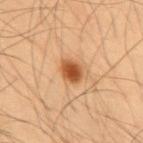Notes:
- biopsy status — no biopsy performed (imaged during a skin exam)
- patient — male, aged around 55
- size — about 2.5 mm
- illumination — cross-polarized illumination
- automated metrics — an outline eccentricity of about 0.6 (0 = round, 1 = elongated) and a shape-asymmetry score of about 0.15 (0 = symmetric); a classifier nevus-likeness of about 100/100 and a lesion-detection confidence of about 100/100
- site — the mid back
- imaging modality — total-body-photography crop, ~15 mm field of view